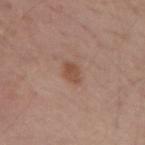This lesion was catalogued during total-body skin photography and was not selected for biopsy. A male patient, in their mid- to late 50s. The lesion is on the right upper arm. A lesion tile, about 15 mm wide, cut from a 3D total-body photograph. About 3 mm across.